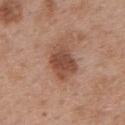{"biopsy_status": "not biopsied; imaged during a skin examination", "patient": {"sex": "female", "age_approx": 40}, "lesion_size": {"long_diameter_mm_approx": 4.0}, "image": {"source": "total-body photography crop", "field_of_view_mm": 15}, "site": "back", "lighting": "white-light"}